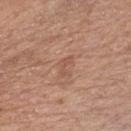Assessment:
This lesion was catalogued during total-body skin photography and was not selected for biopsy.
Clinical summary:
Captured under white-light illumination. The lesion is located on the chest. The patient is a male about 70 years old. A 15 mm crop from a total-body photograph taken for skin-cancer surveillance. Longest diameter approximately 2.5 mm.